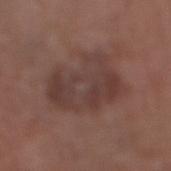Impression: Part of a total-body skin-imaging series; this lesion was reviewed on a skin check and was not flagged for biopsy. Image and clinical context: A 15 mm close-up extracted from a 3D total-body photography capture. Measured at roughly 8.5 mm in maximum diameter. A female patient, aged 78 to 82. The tile uses white-light illumination. On the right lower leg. The lesion-visualizer software estimated a lesion area of about 27 mm², an eccentricity of roughly 0.85, and two-axis asymmetry of about 0.25. The software also gave an average lesion color of about L≈38 a*≈18 b*≈21 (CIELAB), about 7 CIELAB-L* units darker than the surrounding skin, and a normalized lesion–skin contrast near 6.5. The software also gave a border-irregularity index near 4/10, a color-variation rating of about 3.5/10, and radial color variation of about 1. The software also gave an automated nevus-likeness rating near 0 out of 100 and a lesion-detection confidence of about 100/100.